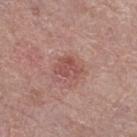* workup · no biopsy performed (imaged during a skin exam)
* image source · total-body-photography crop, ~15 mm field of view
* location · the leg
* subject · male, in their mid-70s
* illumination · white-light